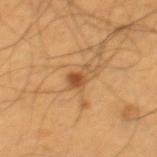Assessment: Recorded during total-body skin imaging; not selected for excision or biopsy. Acquisition and patient details: Located on the leg. An algorithmic analysis of the crop reported a lesion color around L≈50 a*≈22 b*≈39 in CIELAB, a lesion–skin lightness drop of about 10, and a normalized border contrast of about 7.5. The software also gave an automated nevus-likeness rating near 85 out of 100 and lesion-presence confidence of about 100/100. About 3 mm across. The patient is a male roughly 55 years of age. A 15 mm crop from a total-body photograph taken for skin-cancer surveillance.